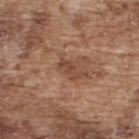Clinical impression:
Part of a total-body skin-imaging series; this lesion was reviewed on a skin check and was not flagged for biopsy.
Clinical summary:
Cropped from a whole-body photographic skin survey; the tile spans about 15 mm. The lesion is located on the upper back. A male patient, about 75 years old. Imaged with white-light lighting. Longest diameter approximately 3 mm.